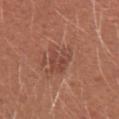Part of a total-body skin-imaging series; this lesion was reviewed on a skin check and was not flagged for biopsy. The lesion's longest dimension is about 4 mm. A female patient in their mid- to late 20s. A roughly 15 mm field-of-view crop from a total-body skin photograph. Located on the right upper arm.